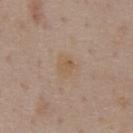Assessment: Captured during whole-body skin photography for melanoma surveillance; the lesion was not biopsied. Acquisition and patient details: This is a white-light tile. The total-body-photography lesion software estimated a footprint of about 4.5 mm² and a shape eccentricity near 0.6. The analysis additionally found a border-irregularity rating of about 3/10, a color-variation rating of about 2/10, and radial color variation of about 1. A male patient, aged approximately 60. The lesion is on the chest. This image is a 15 mm lesion crop taken from a total-body photograph.